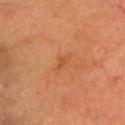{
  "biopsy_status": "not biopsied; imaged during a skin examination",
  "automated_metrics": {
    "eccentricity": 0.85,
    "shape_asymmetry": 0.4,
    "cielab_L": 46,
    "cielab_a": 25,
    "cielab_b": 37,
    "vs_skin_darker_L": 7.0,
    "border_irregularity_0_10": 3.5,
    "color_variation_0_10": 0.0,
    "lesion_detection_confidence_0_100": 100
  },
  "image": {
    "source": "total-body photography crop",
    "field_of_view_mm": 15
  },
  "patient": {
    "sex": "male",
    "age_approx": 60
  },
  "site": "head or neck",
  "lesion_size": {
    "long_diameter_mm_approx": 1.5
  },
  "lighting": "cross-polarized"
}